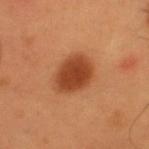Case summary:
- follow-up — total-body-photography surveillance lesion; no biopsy
- patient — male, approximately 55 years of age
- lesion diameter — ≈5 mm
- TBP lesion metrics — an area of roughly 13 mm² and an eccentricity of roughly 0.65; a detector confidence of about 100 out of 100 that the crop contains a lesion
- lighting — cross-polarized
- location — the upper back
- image — total-body-photography crop, ~15 mm field of view